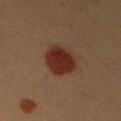Clinical impression: Imaged during a routine full-body skin examination; the lesion was not biopsied and no histopathology is available. Clinical summary: A female patient, aged 38–42. A region of skin cropped from a whole-body photographic capture, roughly 15 mm wide. Automated tile analysis of the lesion measured an area of roughly 11 mm², an outline eccentricity of about 0.55 (0 = round, 1 = elongated), and a shape-asymmetry score of about 0.15 (0 = symmetric). It also reported an automated nevus-likeness rating near 100 out of 100 and lesion-presence confidence of about 100/100. This is a cross-polarized tile. On the right upper arm.